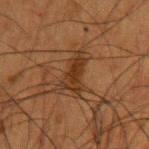Clinical impression:
Captured during whole-body skin photography for melanoma surveillance; the lesion was not biopsied.
Context:
From the left upper arm. A male patient in their 50s. A roughly 15 mm field-of-view crop from a total-body skin photograph.